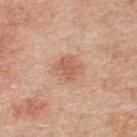| key | value |
|---|---|
| workup | total-body-photography surveillance lesion; no biopsy |
| automated lesion analysis | an outline eccentricity of about 0.5 (0 = round, 1 = elongated) and two-axis asymmetry of about 0.25; a lesion color around L≈59 a*≈23 b*≈32 in CIELAB, roughly 9 lightness units darker than nearby skin, and a normalized border contrast of about 6; a border-irregularity index near 3/10, internal color variation of about 2 on a 0–10 scale, and a peripheral color-asymmetry measure near 0.5; a nevus-likeness score of about 45/100 and a detector confidence of about 100 out of 100 that the crop contains a lesion |
| size | ~2.5 mm (longest diameter) |
| lighting | white-light illumination |
| subject | male, aged around 70 |
| body site | the upper back |
| imaging modality | total-body-photography crop, ~15 mm field of view |